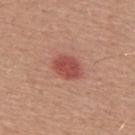Part of a total-body skin-imaging series; this lesion was reviewed on a skin check and was not flagged for biopsy. The subject is a male aged 53–57. This is a white-light tile. A 15 mm close-up tile from a total-body photography series done for melanoma screening. Located on the upper back. About 3.5 mm across.